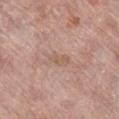The lesion was tiled from a total-body skin photograph and was not biopsied.
The lesion-visualizer software estimated a mean CIELAB color near L≈58 a*≈19 b*≈27 and about 6 CIELAB-L* units darker than the surrounding skin. And it measured a border-irregularity index near 2.5/10, a color-variation rating of about 0.5/10, and radial color variation of about 0. It also reported a classifier nevus-likeness of about 0/100.
A female patient, in their 60s.
Imaged with white-light lighting.
A 15 mm close-up extracted from a 3D total-body photography capture.
From the right lower leg.
Longest diameter approximately 3 mm.